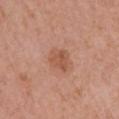Recorded during total-body skin imaging; not selected for excision or biopsy.
The lesion is on the left upper arm.
A female patient about 50 years old.
Approximately 3 mm at its widest.
Imaged with white-light lighting.
A close-up tile cropped from a whole-body skin photograph, about 15 mm across.